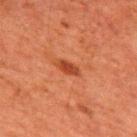notes = catalogued during a skin exam; not biopsied
body site = the upper back
tile lighting = cross-polarized
automated lesion analysis = a lesion color around L≈35 a*≈28 b*≈32 in CIELAB, a lesion–skin lightness drop of about 9, and a normalized border contrast of about 8; border irregularity of about 2.5 on a 0–10 scale; a nevus-likeness score of about 85/100 and lesion-presence confidence of about 100/100
subject = male, aged approximately 60
imaging modality = ~15 mm tile from a whole-body skin photo